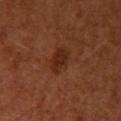{
  "biopsy_status": "not biopsied; imaged during a skin examination",
  "image": {
    "source": "total-body photography crop",
    "field_of_view_mm": 15
  },
  "patient": {
    "sex": "female",
    "age_approx": 65
  },
  "site": "right upper arm"
}